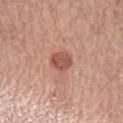Impression: Imaged during a routine full-body skin examination; the lesion was not biopsied and no histopathology is available. Context: Located on the left forearm. The subject is a female aged 58–62. The tile uses white-light illumination. A 15 mm close-up extracted from a 3D total-body photography capture. Automated image analysis of the tile measured border irregularity of about 1.5 on a 0–10 scale, a color-variation rating of about 2.5/10, and radial color variation of about 1. The analysis additionally found a detector confidence of about 100 out of 100 that the crop contains a lesion.